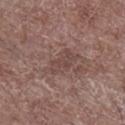Captured during whole-body skin photography for melanoma surveillance; the lesion was not biopsied.
Located on the right lower leg.
This is a white-light tile.
Cropped from a whole-body photographic skin survey; the tile spans about 15 mm.
A male patient aged approximately 70.
Automated tile analysis of the lesion measured a lesion color around L≈44 a*≈18 b*≈21 in CIELAB and a normalized border contrast of about 5.5. It also reported a border-irregularity index near 4/10, internal color variation of about 2 on a 0–10 scale, and radial color variation of about 0.5.